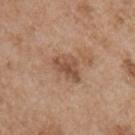biopsy_status: not biopsied; imaged during a skin examination
image:
  source: total-body photography crop
  field_of_view_mm: 15
automated_metrics:
  border_irregularity_0_10: 3.5
  color_variation_0_10: 3.5
  peripheral_color_asymmetry: 1.0
  nevus_likeness_0_100: 0
  lesion_detection_confidence_0_100: 100
site: front of the torso
lesion_size:
  long_diameter_mm_approx: 3.5
patient:
  sex: male
  age_approx: 55